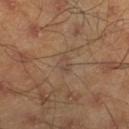biopsy_status: not biopsied; imaged during a skin examination
lighting: cross-polarized
lesion_size:
  long_diameter_mm_approx: 2.5
patient:
  sex: male
  age_approx: 45
site: right lower leg
automated_metrics:
  area_mm2_approx: 3.5
  eccentricity: 0.8
  shape_asymmetry: 0.35
  cielab_L: 45
  cielab_a: 15
  cielab_b: 26
  vs_skin_darker_L: 5.0
  vs_skin_contrast_norm: 5.0
  nevus_likeness_0_100: 0
  lesion_detection_confidence_0_100: 90
image:
  source: total-body photography crop
  field_of_view_mm: 15